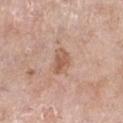follow-up: catalogued during a skin exam; not biopsied
imaging modality: ~15 mm crop, total-body skin-cancer survey
site: the right lower leg
illumination: white-light illumination
subject: female, aged 68–72